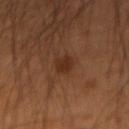patient: male, about 50 years old
TBP lesion metrics: a lesion area of about 4 mm² and two-axis asymmetry of about 0.25; a detector confidence of about 100 out of 100 that the crop contains a lesion
anatomic site: the left forearm
imaging modality: total-body-photography crop, ~15 mm field of view